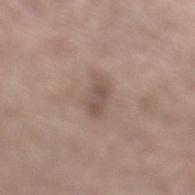Clinical summary:
The recorded lesion diameter is about 3.5 mm. A 15 mm crop from a total-body photograph taken for skin-cancer surveillance. The total-body-photography lesion software estimated a footprint of about 5 mm², an eccentricity of roughly 0.85, and a shape-asymmetry score of about 0.3 (0 = symmetric). A male patient in their mid-60s. This is a white-light tile. The lesion is located on the left lower leg.